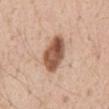  biopsy_status: not biopsied; imaged during a skin examination
  patient:
    sex: male
    age_approx: 55
  automated_metrics:
    vs_skin_contrast_norm: 11.0
    nevus_likeness_0_100: 90
    lesion_detection_confidence_0_100: 100
  site: abdomen
  lighting: white-light
  lesion_size:
    long_diameter_mm_approx: 5.0
  image:
    source: total-body photography crop
    field_of_view_mm: 15